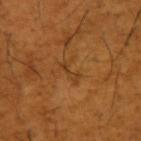Automated image analysis of the tile measured a shape eccentricity near 0.8 and two-axis asymmetry of about 0.55. And it measured a lesion color around L≈40 a*≈22 b*≈39 in CIELAB, a lesion–skin lightness drop of about 6, and a normalized border contrast of about 5. The software also gave a border-irregularity index near 7.5/10 and internal color variation of about 0 on a 0–10 scale.
The lesion is on the left upper arm.
Imaged with cross-polarized lighting.
This image is a 15 mm lesion crop taken from a total-body photograph.
The patient is a male approximately 65 years of age.
Longest diameter approximately 3 mm.